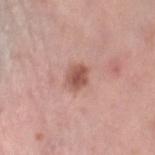The lesion was tiled from a total-body skin photograph and was not biopsied. On the leg. A 15 mm close-up extracted from a 3D total-body photography capture. This is a white-light tile. Automated image analysis of the tile measured a footprint of about 5.5 mm², a shape eccentricity near 0.55, and a symmetry-axis asymmetry near 0.15. And it measured a border-irregularity index near 1.5/10 and radial color variation of about 1. The analysis additionally found a nevus-likeness score of about 80/100. A female patient aged approximately 40. The lesion's longest dimension is about 3 mm.